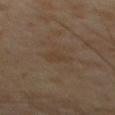workup: no biopsy performed (imaged during a skin exam)
subject: male, aged 63–67
automated lesion analysis: a border-irregularity index near 4/10 and peripheral color asymmetry of about 0.5
lesion diameter: ~2.5 mm (longest diameter)
image source: ~15 mm tile from a whole-body skin photo